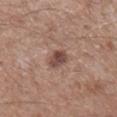Case summary:
- biopsy status · total-body-photography surveillance lesion; no biopsy